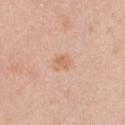Q: Is there a histopathology result?
A: no biopsy performed (imaged during a skin exam)
Q: Who is the patient?
A: female, in their mid- to late 40s
Q: What is the lesion's diameter?
A: ≈2.5 mm
Q: Where on the body is the lesion?
A: the chest
Q: How was the tile lit?
A: white-light
Q: How was this image acquired?
A: ~15 mm crop, total-body skin-cancer survey